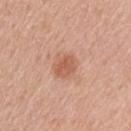workup: catalogued during a skin exam; not biopsied
lighting: white-light
patient: male, aged around 65
image-analysis metrics: a footprint of about 8 mm² and a shape-asymmetry score of about 0.2 (0 = symmetric); an average lesion color of about L≈59 a*≈23 b*≈32 (CIELAB) and a normalized lesion–skin contrast near 6.5; a border-irregularity index near 1.5/10, internal color variation of about 3 on a 0–10 scale, and a peripheral color-asymmetry measure near 1; a nevus-likeness score of about 80/100 and lesion-presence confidence of about 100/100
site: the upper back
diameter: ≈3.5 mm
image: ~15 mm tile from a whole-body skin photo